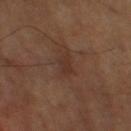Captured during whole-body skin photography for melanoma surveillance; the lesion was not biopsied. Cropped from a whole-body photographic skin survey; the tile spans about 15 mm. The lesion is on the left leg. An algorithmic analysis of the crop reported a lesion area of about 3.5 mm², an outline eccentricity of about 0.9 (0 = round, 1 = elongated), and a symmetry-axis asymmetry near 0.45. It also reported a lesion–skin lightness drop of about 5. A male subject aged 63 to 67. Approximately 3 mm at its widest. Captured under cross-polarized illumination.